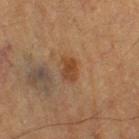biopsy_status: not biopsied; imaged during a skin examination
image:
  source: total-body photography crop
  field_of_view_mm: 15
patient:
  sex: male
  age_approx: 85+
site: left thigh
lesion_size:
  long_diameter_mm_approx: 3.0
automated_metrics:
  eccentricity: 0.75
  shape_asymmetry: 0.2
  nevus_likeness_0_100: 90